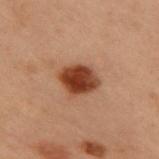Automated tile analysis of the lesion measured an area of roughly 9.5 mm², an eccentricity of roughly 0.45, and two-axis asymmetry of about 0.15. The analysis additionally found a lesion color around L≈33 a*≈22 b*≈28 in CIELAB. The software also gave an automated nevus-likeness rating near 100 out of 100 and a lesion-detection confidence of about 100/100.
A roughly 15 mm field-of-view crop from a total-body skin photograph.
The patient is a male approximately 55 years of age.
The lesion is on the upper back.
Longest diameter approximately 3.5 mm.
Imaged with cross-polarized lighting.